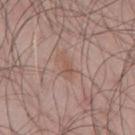lighting — white-light illumination
body site — the mid back
TBP lesion metrics — a footprint of about 3 mm² and two-axis asymmetry of about 0.45; a lesion color around L≈53 a*≈19 b*≈26 in CIELAB, roughly 6 lightness units darker than nearby skin, and a lesion-to-skin contrast of about 5.5 (normalized; higher = more distinct)
subject — male, aged 43–47
lesion diameter — ~3 mm (longest diameter)
image — 15 mm crop, total-body photography